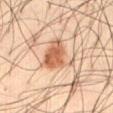Assessment: Captured during whole-body skin photography for melanoma surveillance; the lesion was not biopsied. Image and clinical context: From the front of the torso. The patient is a male aged 33–37. A close-up tile cropped from a whole-body skin photograph, about 15 mm across.